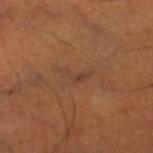biopsy status: total-body-photography surveillance lesion; no biopsy | image source: 15 mm crop, total-body photography | illumination: cross-polarized | patient: male, aged approximately 50 | site: the leg | image-analysis metrics: an average lesion color of about L≈39 a*≈19 b*≈28 (CIELAB) and about 6 CIELAB-L* units darker than the surrounding skin | size: about 3 mm.